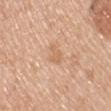The lesion was tiled from a total-body skin photograph and was not biopsied.
Captured under white-light illumination.
This image is a 15 mm lesion crop taken from a total-body photograph.
The lesion is located on the upper back.
The total-body-photography lesion software estimated a lesion area of about 3.5 mm², a shape eccentricity near 0.8, and a shape-asymmetry score of about 0.5 (0 = symmetric). The analysis additionally found roughly 7 lightness units darker than nearby skin and a normalized lesion–skin contrast near 5. The analysis additionally found a border-irregularity rating of about 5/10, a within-lesion color-variation index near 0.5/10, and radial color variation of about 0. The software also gave an automated nevus-likeness rating near 0 out of 100.
A male subject, roughly 50 years of age.